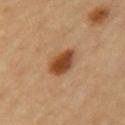The patient is a female aged around 60. Located on the arm. Imaged with cross-polarized lighting. A region of skin cropped from a whole-body photographic capture, roughly 15 mm wide.